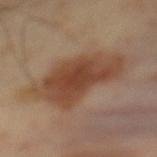Imaged during a routine full-body skin examination; the lesion was not biopsied and no histopathology is available. A male patient aged 38 to 42. The total-body-photography lesion software estimated a lesion area of about 29 mm², an eccentricity of roughly 0.85, and two-axis asymmetry of about 0.25. The software also gave a mean CIELAB color near L≈44 a*≈20 b*≈30, roughly 10 lightness units darker than nearby skin, and a normalized border contrast of about 8.5. And it measured an automated nevus-likeness rating near 95 out of 100 and lesion-presence confidence of about 100/100. A lesion tile, about 15 mm wide, cut from a 3D total-body photograph. Imaged with cross-polarized lighting. The lesion is located on the mid back. The recorded lesion diameter is about 8.5 mm.